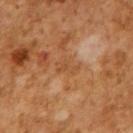Part of a total-body skin-imaging series; this lesion was reviewed on a skin check and was not flagged for biopsy.
The total-body-photography lesion software estimated a border-irregularity rating of about 4/10 and internal color variation of about 2 on a 0–10 scale. The software also gave a nevus-likeness score of about 0/100 and a lesion-detection confidence of about 95/100.
The tile uses cross-polarized illumination.
A 15 mm crop from a total-body photograph taken for skin-cancer surveillance.
A male subject, in their mid-60s.
Longest diameter approximately 3 mm.